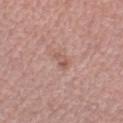{"biopsy_status": "not biopsied; imaged during a skin examination", "image": {"source": "total-body photography crop", "field_of_view_mm": 15}, "site": "right lower leg", "lighting": "white-light", "patient": {"sex": "female", "age_approx": 40}}